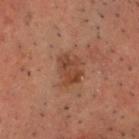follow-up=no biopsy performed (imaged during a skin exam); site=the head or neck; illumination=cross-polarized; patient=male, roughly 50 years of age; imaging modality=15 mm crop, total-body photography.